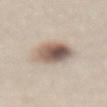No biopsy was performed on this lesion — it was imaged during a full skin examination and was not determined to be concerning.
A female patient, approximately 60 years of age.
A 15 mm crop from a total-body photograph taken for skin-cancer surveillance.
The lesion is on the front of the torso.